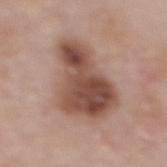Image and clinical context:
The lesion-visualizer software estimated an area of roughly 27 mm², an eccentricity of roughly 0.7, and two-axis asymmetry of about 0.45. And it measured a classifier nevus-likeness of about 10/100. The subject is a female about 50 years old. The lesion is on the upper back. A close-up tile cropped from a whole-body skin photograph, about 15 mm across. About 6.5 mm across.